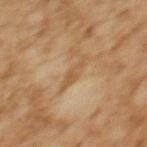Cropped from a total-body skin-imaging series; the visible field is about 15 mm. The lesion is on the upper back. A female patient, about 60 years old. Longest diameter approximately 4 mm.